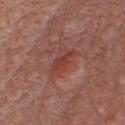Q: Was a biopsy performed?
A: imaged on a skin check; not biopsied
Q: Lesion location?
A: the front of the torso
Q: Who is the patient?
A: male, in their mid- to late 70s
Q: What is the imaging modality?
A: total-body-photography crop, ~15 mm field of view
Q: How was the tile lit?
A: white-light illumination